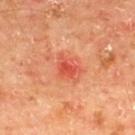Recorded during total-body skin imaging; not selected for excision or biopsy.